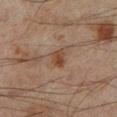Notes:
* biopsy status: total-body-photography surveillance lesion; no biopsy
* location: the left lower leg
* lighting: cross-polarized
* subject: male, aged around 45
* image source: 15 mm crop, total-body photography
* automated lesion analysis: a peripheral color-asymmetry measure near 1; lesion-presence confidence of about 100/100